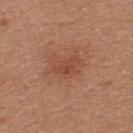Captured during whole-body skin photography for melanoma surveillance; the lesion was not biopsied. The lesion is on the back. The lesion's longest dimension is about 3 mm. Automated image analysis of the tile measured roughly 7 lightness units darker than nearby skin and a normalized border contrast of about 5. And it measured a border-irregularity index near 3.5/10, a within-lesion color-variation index near 2/10, and a peripheral color-asymmetry measure near 0.5. The analysis additionally found an automated nevus-likeness rating near 0 out of 100 and a detector confidence of about 100 out of 100 that the crop contains a lesion. A female subject, aged approximately 25. Captured under white-light illumination. A region of skin cropped from a whole-body photographic capture, roughly 15 mm wide.